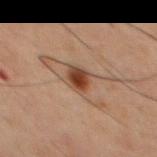workup: no biopsy performed (imaged during a skin exam) | subject: male, approximately 60 years of age | imaging modality: total-body-photography crop, ~15 mm field of view | size: ≈3 mm | illumination: cross-polarized | anatomic site: the front of the torso.